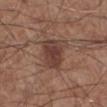biopsy status = catalogued during a skin exam; not biopsied
patient = male, aged 58–62
acquisition = ~15 mm crop, total-body skin-cancer survey
location = the right lower leg